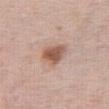Part of a total-body skin-imaging series; this lesion was reviewed on a skin check and was not flagged for biopsy. A lesion tile, about 15 mm wide, cut from a 3D total-body photograph. Captured under white-light illumination. Longest diameter approximately 3.5 mm. Automated image analysis of the tile measured a footprint of about 7.5 mm². The software also gave an average lesion color of about L≈56 a*≈20 b*≈28 (CIELAB) and a lesion-to-skin contrast of about 9 (normalized; higher = more distinct). The software also gave a border-irregularity index near 2.5/10 and peripheral color asymmetry of about 1. The software also gave an automated nevus-likeness rating near 90 out of 100 and a detector confidence of about 100 out of 100 that the crop contains a lesion. Located on the abdomen. A female patient approximately 65 years of age.